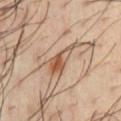No biopsy was performed on this lesion — it was imaged during a full skin examination and was not determined to be concerning.
The tile uses cross-polarized illumination.
Approximately 4 mm at its widest.
Automated tile analysis of the lesion measured an area of roughly 6 mm², an eccentricity of roughly 0.9, and a symmetry-axis asymmetry near 0.45. It also reported a mean CIELAB color near L≈54 a*≈19 b*≈31, roughly 11 lightness units darker than nearby skin, and a normalized border contrast of about 8. The analysis additionally found radial color variation of about 2. The analysis additionally found an automated nevus-likeness rating near 60 out of 100 and a lesion-detection confidence of about 100/100.
A 15 mm crop from a total-body photograph taken for skin-cancer surveillance.
On the chest.
A male patient, in their 40s.